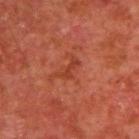Q: Was a biopsy performed?
A: catalogued during a skin exam; not biopsied
Q: What kind of image is this?
A: 15 mm crop, total-body photography
Q: Automated lesion metrics?
A: a footprint of about 3 mm², an outline eccentricity of about 0.9 (0 = round, 1 = elongated), and two-axis asymmetry of about 0.5; a border-irregularity rating of about 6/10 and internal color variation of about 0 on a 0–10 scale; a nevus-likeness score of about 0/100 and a detector confidence of about 100 out of 100 that the crop contains a lesion
Q: Lesion location?
A: the back
Q: Patient demographics?
A: male, about 65 years old